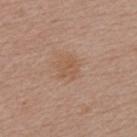Notes:
* notes: no biopsy performed (imaged during a skin exam)
* diameter: about 2.5 mm
* site: the left upper arm
* automated metrics: an area of roughly 4.5 mm², a shape eccentricity near 0.5, and a symmetry-axis asymmetry near 0.3; a lesion color around L≈55 a*≈19 b*≈31 in CIELAB, roughly 6 lightness units darker than nearby skin, and a normalized lesion–skin contrast near 5; border irregularity of about 3.5 on a 0–10 scale, internal color variation of about 2.5 on a 0–10 scale, and a peripheral color-asymmetry measure near 1; lesion-presence confidence of about 100/100
* tile lighting: white-light
* imaging modality: 15 mm crop, total-body photography
* patient: female, in their mid-40s